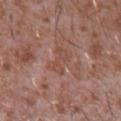Case summary:
• notes · imaged on a skin check; not biopsied
• lesion diameter · about 3.5 mm
• body site · the chest
• subject · male, aged 43–47
• image-analysis metrics · a lesion area of about 4.5 mm², a shape eccentricity near 0.9, and a symmetry-axis asymmetry near 0.4; a border-irregularity index near 6/10 and peripheral color asymmetry of about 0; an automated nevus-likeness rating near 0 out of 100 and a detector confidence of about 85 out of 100 that the crop contains a lesion
• imaging modality · ~15 mm tile from a whole-body skin photo